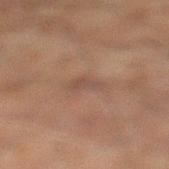workup — imaged on a skin check; not biopsied | imaging modality — ~15 mm tile from a whole-body skin photo | patient — male, in their 60s | tile lighting — cross-polarized illumination | site — the left lower leg | lesion size — ~2.5 mm (longest diameter).